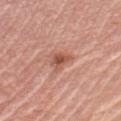workup = imaged on a skin check; not biopsied | automated metrics = a lesion area of about 3.5 mm², an eccentricity of roughly 0.75, and two-axis asymmetry of about 0.4; an average lesion color of about L≈53 a*≈26 b*≈30 (CIELAB), about 11 CIELAB-L* units darker than the surrounding skin, and a normalized border contrast of about 7.5; a border-irregularity index near 4/10 and a within-lesion color-variation index near 2.5/10; a lesion-detection confidence of about 100/100 | acquisition = 15 mm crop, total-body photography | illumination = white-light illumination | patient = female, about 65 years old | body site = the arm | size = ≈2.5 mm.